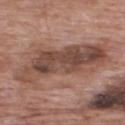<record>
  <biopsy_status>not biopsied; imaged during a skin examination</biopsy_status>
  <lesion_size>
    <long_diameter_mm_approx>10.5</long_diameter_mm_approx>
  </lesion_size>
  <automated_metrics>
    <border_irregularity_0_10>5.0</border_irregularity_0_10>
    <peripheral_color_asymmetry>2.5</peripheral_color_asymmetry>
  </automated_metrics>
  <image>
    <source>total-body photography crop</source>
    <field_of_view_mm>15</field_of_view_mm>
  </image>
  <site>upper back</site>
  <lighting>white-light</lighting>
  <patient>
    <sex>male</sex>
    <age_approx>70</age_approx>
  </patient>
</record>